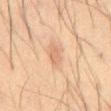<case>
  <biopsy_status>not biopsied; imaged during a skin examination</biopsy_status>
  <site>front of the torso</site>
  <image>
    <source>total-body photography crop</source>
    <field_of_view_mm>15</field_of_view_mm>
  </image>
  <lighting>cross-polarized</lighting>
  <lesion_size>
    <long_diameter_mm_approx>2.5</long_diameter_mm_approx>
  </lesion_size>
  <patient>
    <sex>male</sex>
    <age_approx>60</age_approx>
  </patient>
</case>